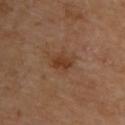Part of a total-body skin-imaging series; this lesion was reviewed on a skin check and was not flagged for biopsy.
The tile uses cross-polarized illumination.
A close-up tile cropped from a whole-body skin photograph, about 15 mm across.
Located on the upper back.
The recorded lesion diameter is about 3 mm.